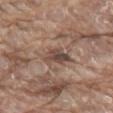This lesion was catalogued during total-body skin photography and was not selected for biopsy. Cropped from a total-body skin-imaging series; the visible field is about 15 mm. A male patient approximately 80 years of age. The lesion is located on the abdomen.